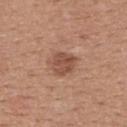{"biopsy_status": "not biopsied; imaged during a skin examination", "lesion_size": {"long_diameter_mm_approx": 3.5}, "site": "upper back", "image": {"source": "total-body photography crop", "field_of_view_mm": 15}, "patient": {"sex": "female", "age_approx": 35}}